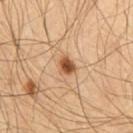Case summary:
* workup · total-body-photography surveillance lesion; no biopsy
* anatomic site · the chest
* lighting · cross-polarized
* patient · male, in their mid- to late 60s
* imaging modality · 15 mm crop, total-body photography
* diameter · about 2.5 mm